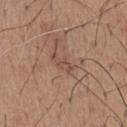Q: Was a biopsy performed?
A: no biopsy performed (imaged during a skin exam)
Q: Lesion location?
A: the chest
Q: How large is the lesion?
A: ~3 mm (longest diameter)
Q: What are the patient's age and sex?
A: male, aged 63–67
Q: What kind of image is this?
A: ~15 mm tile from a whole-body skin photo
Q: What lighting was used for the tile?
A: white-light illumination